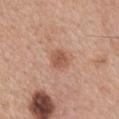Part of a total-body skin-imaging series; this lesion was reviewed on a skin check and was not flagged for biopsy. A roughly 15 mm field-of-view crop from a total-body skin photograph. The lesion's longest dimension is about 2.5 mm. The lesion is located on the mid back. A male patient, approximately 55 years of age. Captured under white-light illumination.